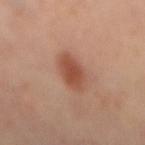<lesion>
  <biopsy_status>not biopsied; imaged during a skin examination</biopsy_status>
  <lesion_size>
    <long_diameter_mm_approx>5.0</long_diameter_mm_approx>
  </lesion_size>
  <patient>
    <sex>male</sex>
    <age_approx>55</age_approx>
  </patient>
  <automated_metrics>
    <cielab_L>50</cielab_L>
    <cielab_a>24</cielab_a>
    <cielab_b>31</cielab_b>
    <vs_skin_darker_L>11.0</vs_skin_darker_L>
    <vs_skin_contrast_norm>8.0</vs_skin_contrast_norm>
    <nevus_likeness_0_100>95</nevus_likeness_0_100>
    <lesion_detection_confidence_0_100>100</lesion_detection_confidence_0_100>
  </automated_metrics>
  <image>
    <source>total-body photography crop</source>
    <field_of_view_mm>15</field_of_view_mm>
  </image>
  <site>mid back</site>
</lesion>